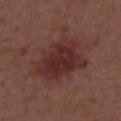biopsy_status: not biopsied; imaged during a skin examination
patient:
  sex: male
  age_approx: 55
image:
  source: total-body photography crop
  field_of_view_mm: 15
automated_metrics:
  cielab_L: 30
  cielab_a: 22
  cielab_b: 21
  vs_skin_contrast_norm: 8.5
  border_irregularity_0_10: 3.0
  color_variation_0_10: 3.0
  nevus_likeness_0_100: 70
  lesion_detection_confidence_0_100: 100
lighting: white-light
site: upper back
lesion_size:
  long_diameter_mm_approx: 7.0